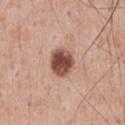| field | value |
|---|---|
| follow-up | catalogued during a skin exam; not biopsied |
| automated lesion analysis | an outline eccentricity of about 0.55 (0 = round, 1 = elongated) and two-axis asymmetry of about 0.1; a lesion color around L≈49 a*≈22 b*≈26 in CIELAB, roughly 18 lightness units darker than nearby skin, and a lesion-to-skin contrast of about 12 (normalized; higher = more distinct); a detector confidence of about 100 out of 100 that the crop contains a lesion |
| body site | the chest |
| lighting | white-light |
| subject | male, approximately 55 years of age |
| diameter | ≈3.5 mm |
| image source | 15 mm crop, total-body photography |